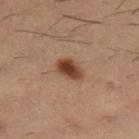Q: Was this lesion biopsied?
A: no biopsy performed (imaged during a skin exam)
Q: Who is the patient?
A: male, roughly 30 years of age
Q: What is the lesion's diameter?
A: ≈3.5 mm
Q: Illumination type?
A: cross-polarized illumination
Q: What kind of image is this?
A: 15 mm crop, total-body photography
Q: What is the anatomic site?
A: the right lower leg